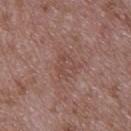<lesion>
<biopsy_status>not biopsied; imaged during a skin examination</biopsy_status>
<image>
  <source>total-body photography crop</source>
  <field_of_view_mm>15</field_of_view_mm>
</image>
<site>upper back</site>
<patient>
  <sex>male</sex>
  <age_approx>50</age_approx>
</patient>
<automated_metrics>
  <border_irregularity_0_10>5.0</border_irregularity_0_10>
  <peripheral_color_asymmetry>1.0</peripheral_color_asymmetry>
</automated_metrics>
</lesion>